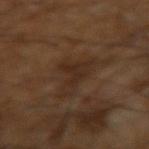Recorded during total-body skin imaging; not selected for excision or biopsy. Automated tile analysis of the lesion measured a lesion area of about 8 mm² and a shape eccentricity near 0.8. The analysis additionally found a mean CIELAB color near L≈26 a*≈15 b*≈24, roughly 6 lightness units darker than nearby skin, and a normalized lesion–skin contrast near 6.5. The software also gave border irregularity of about 7 on a 0–10 scale, internal color variation of about 3 on a 0–10 scale, and peripheral color asymmetry of about 1. And it measured a nevus-likeness score of about 0/100. The patient is a male aged 58–62. The lesion is on the arm. Approximately 4.5 mm at its widest. A 15 mm close-up tile from a total-body photography series done for melanoma screening.